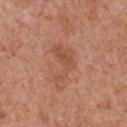Part of a total-body skin-imaging series; this lesion was reviewed on a skin check and was not flagged for biopsy.
From the chest.
A lesion tile, about 15 mm wide, cut from a 3D total-body photograph.
The patient is a male in their mid-60s.
Longest diameter approximately 5.5 mm.
Captured under white-light illumination.